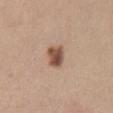{
  "biopsy_status": "not biopsied; imaged during a skin examination",
  "lesion_size": {
    "long_diameter_mm_approx": 3.0
  },
  "image": {
    "source": "total-body photography crop",
    "field_of_view_mm": 15
  },
  "patient": {
    "sex": "female",
    "age_approx": 40
  },
  "lighting": "white-light",
  "site": "mid back"
}